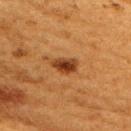Imaged during a routine full-body skin examination; the lesion was not biopsied and no histopathology is available. Imaged with cross-polarized lighting. The patient is a female aged approximately 50. This image is a 15 mm lesion crop taken from a total-body photograph. On the upper back.